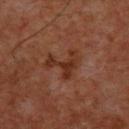notes: catalogued during a skin exam; not biopsied
anatomic site: the chest
diameter: about 4 mm
acquisition: ~15 mm crop, total-body skin-cancer survey
subject: male, approximately 60 years of age
tile lighting: cross-polarized illumination